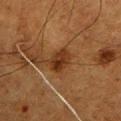No biopsy was performed on this lesion — it was imaged during a full skin examination and was not determined to be concerning. Longest diameter approximately 3.5 mm. The subject is a male aged 48–52. The lesion-visualizer software estimated a lesion area of about 6.5 mm², a shape eccentricity near 0.75, and two-axis asymmetry of about 0.25. The analysis additionally found a mean CIELAB color near L≈27 a*≈19 b*≈28 and a lesion-to-skin contrast of about 9 (normalized; higher = more distinct). It also reported a border-irregularity rating of about 2.5/10 and peripheral color asymmetry of about 2. Cropped from a whole-body photographic skin survey; the tile spans about 15 mm. From the left upper arm. Captured under cross-polarized illumination.